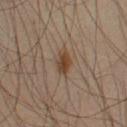Q: Is there a histopathology result?
A: total-body-photography surveillance lesion; no biopsy
Q: Lesion size?
A: ≈3 mm
Q: Automated lesion metrics?
A: an area of roughly 4 mm² and a shape eccentricity near 0.75; a border-irregularity rating of about 2/10, a color-variation rating of about 3.5/10, and radial color variation of about 1; an automated nevus-likeness rating near 95 out of 100 and a lesion-detection confidence of about 100/100
Q: How was the tile lit?
A: cross-polarized
Q: Who is the patient?
A: male, about 40 years old
Q: What is the imaging modality?
A: 15 mm crop, total-body photography
Q: Lesion location?
A: the left upper arm